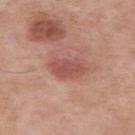Clinical impression:
No biopsy was performed on this lesion — it was imaged during a full skin examination and was not determined to be concerning.
Image and clinical context:
Imaged with white-light lighting. On the right upper arm. The recorded lesion diameter is about 4 mm. A lesion tile, about 15 mm wide, cut from a 3D total-body photograph. An algorithmic analysis of the crop reported border irregularity of about 2 on a 0–10 scale and a peripheral color-asymmetry measure near 1. It also reported a classifier nevus-likeness of about 25/100 and a lesion-detection confidence of about 100/100. A male patient aged around 55.